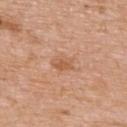Case summary:
* workup: total-body-photography surveillance lesion; no biopsy
* lesion size: ≈2.5 mm
* image-analysis metrics: a lesion area of about 4 mm², a shape eccentricity near 0.7, and a symmetry-axis asymmetry near 0.25; a lesion color around L≈58 a*≈23 b*≈35 in CIELAB, roughly 8 lightness units darker than nearby skin, and a normalized border contrast of about 6
* image: 15 mm crop, total-body photography
* subject: male, aged around 60
* location: the upper back
* illumination: white-light